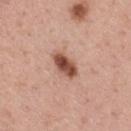biopsy status=catalogued during a skin exam; not biopsied
acquisition=15 mm crop, total-body photography
patient=male, aged 38 to 42
image-analysis metrics=a lesion area of about 6 mm² and a symmetry-axis asymmetry near 0.25; a lesion color around L≈50 a*≈24 b*≈29 in CIELAB, about 17 CIELAB-L* units darker than the surrounding skin, and a normalized border contrast of about 11
anatomic site=the mid back